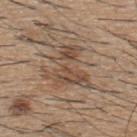Part of a total-body skin-imaging series; this lesion was reviewed on a skin check and was not flagged for biopsy.
This is a white-light tile.
A male patient in their 60s.
The lesion is on the upper back.
About 5.5 mm across.
Cropped from a whole-body photographic skin survey; the tile spans about 15 mm.
Automated tile analysis of the lesion measured a footprint of about 15 mm² and an eccentricity of roughly 0.6. And it measured a lesion color around L≈48 a*≈16 b*≈28 in CIELAB, a lesion–skin lightness drop of about 8, and a lesion-to-skin contrast of about 6.5 (normalized; higher = more distinct). And it measured a detector confidence of about 85 out of 100 that the crop contains a lesion.